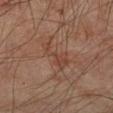Case summary:
* notes — catalogued during a skin exam; not biopsied
* body site — the left forearm
* image — total-body-photography crop, ~15 mm field of view
* patient — male, approximately 45 years of age